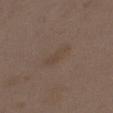workup: no biopsy performed (imaged during a skin exam)
imaging modality: 15 mm crop, total-body photography
body site: the front of the torso
lighting: white-light illumination
patient: female, in their mid-30s
lesion size: ~4 mm (longest diameter)